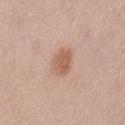Imaged during a routine full-body skin examination; the lesion was not biopsied and no histopathology is available. The lesion is on the left thigh. A female patient aged around 40. The total-body-photography lesion software estimated about 10 CIELAB-L* units darker than the surrounding skin and a normalized border contrast of about 7. A 15 mm crop from a total-body photograph taken for skin-cancer surveillance. Imaged with white-light lighting.